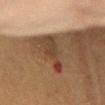No biopsy was performed on this lesion — it was imaged during a full skin examination and was not determined to be concerning.